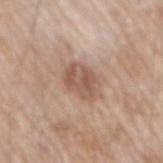| field | value |
|---|---|
| notes | no biopsy performed (imaged during a skin exam) |
| patient | male, aged around 80 |
| TBP lesion metrics | a border-irregularity index near 2/10, a within-lesion color-variation index near 4.5/10, and a peripheral color-asymmetry measure near 1.5; a nevus-likeness score of about 30/100 and a detector confidence of about 100 out of 100 that the crop contains a lesion |
| lighting | white-light illumination |
| anatomic site | the mid back |
| acquisition | 15 mm crop, total-body photography |